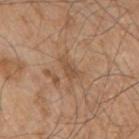Captured under white-light illumination.
The lesion is located on the right upper arm.
An algorithmic analysis of the crop reported an area of roughly 3.5 mm². And it measured a lesion–skin lightness drop of about 7 and a lesion-to-skin contrast of about 5.5 (normalized; higher = more distinct).
A male patient, approximately 80 years of age.
A close-up tile cropped from a whole-body skin photograph, about 15 mm across.